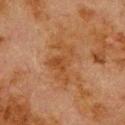Clinical summary:
Cropped from a total-body skin-imaging series; the visible field is about 15 mm. A male subject approximately 80 years of age. Approximately 5 mm at its widest. Located on the back. This is a cross-polarized tile. The total-body-photography lesion software estimated about 6 CIELAB-L* units darker than the surrounding skin and a normalized border contrast of about 6. And it measured a border-irregularity index near 8/10, a color-variation rating of about 3.5/10, and peripheral color asymmetry of about 1.